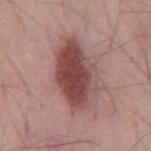Assessment:
Imaged during a routine full-body skin examination; the lesion was not biopsied and no histopathology is available.
Acquisition and patient details:
A male patient in their 60s. The tile uses white-light illumination. A region of skin cropped from a whole-body photographic capture, roughly 15 mm wide. On the mid back. The lesion-visualizer software estimated an automated nevus-likeness rating near 90 out of 100 and lesion-presence confidence of about 100/100. Approximately 7 mm at its widest.